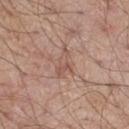Clinical impression:
Imaged during a routine full-body skin examination; the lesion was not biopsied and no histopathology is available.
Clinical summary:
Approximately 4 mm at its widest. Imaged with white-light lighting. A 15 mm close-up extracted from a 3D total-body photography capture. A male subject aged around 55. From the right thigh. Automated image analysis of the tile measured border irregularity of about 6 on a 0–10 scale and radial color variation of about 1. It also reported an automated nevus-likeness rating near 0 out of 100.